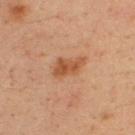A close-up tile cropped from a whole-body skin photograph, about 15 mm across. Automated image analysis of the tile measured border irregularity of about 3.5 on a 0–10 scale, a within-lesion color-variation index near 3/10, and peripheral color asymmetry of about 1. The software also gave a nevus-likeness score of about 65/100 and lesion-presence confidence of about 100/100. From the upper back. The patient is a male in their mid-30s. The tile uses cross-polarized illumination. The lesion's longest dimension is about 3.5 mm.